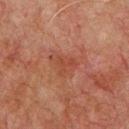<case>
  <biopsy_status>not biopsied; imaged during a skin examination</biopsy_status>
</case>